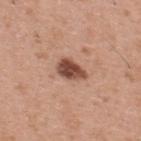Part of a total-body skin-imaging series; this lesion was reviewed on a skin check and was not flagged for biopsy. A male patient, approximately 30 years of age. A close-up tile cropped from a whole-body skin photograph, about 15 mm across. From the upper back.